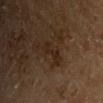workup: catalogued during a skin exam; not biopsied | imaging modality: ~15 mm tile from a whole-body skin photo | subject: male, in their mid- to late 60s | tile lighting: cross-polarized | diameter: about 3.5 mm | site: the front of the torso.